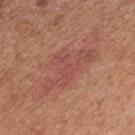<record>
<patient>
  <sex>male</sex>
  <age_approx>55</age_approx>
</patient>
<image>
  <source>total-body photography crop</source>
  <field_of_view_mm>15</field_of_view_mm>
</image>
<site>chest</site>
</record>